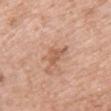From the chest.
A female subject aged 38–42.
The recorded lesion diameter is about 3.5 mm.
A region of skin cropped from a whole-body photographic capture, roughly 15 mm wide.
The lesion-visualizer software estimated a classifier nevus-likeness of about 0/100 and a detector confidence of about 95 out of 100 that the crop contains a lesion.
Captured under white-light illumination.